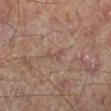subject — male, aged 68 to 72 | automated lesion analysis — an area of roughly 2.5 mm² and an outline eccentricity of about 0.9 (0 = round, 1 = elongated); an automated nevus-likeness rating near 0 out of 100 and a lesion-detection confidence of about 55/100 | imaging modality — ~15 mm crop, total-body skin-cancer survey | lesion size — ~2.5 mm (longest diameter) | anatomic site — the left lower leg | tile lighting — cross-polarized.